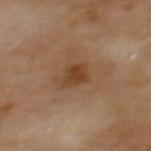Recorded during total-body skin imaging; not selected for excision or biopsy.
On the back.
A 15 mm close-up extracted from a 3D total-body photography capture.
A male patient aged approximately 70.